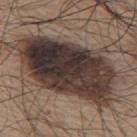notes — no biopsy performed (imaged during a skin exam)
lighting — white-light illumination
image — ~15 mm crop, total-body skin-cancer survey
patient — male, roughly 75 years of age
body site — the mid back
size — ≈12 mm